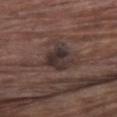Assessment:
Imaged during a routine full-body skin examination; the lesion was not biopsied and no histopathology is available.
Image and clinical context:
A male subject, approximately 80 years of age. The lesion is located on the chest. This image is a 15 mm lesion crop taken from a total-body photograph.